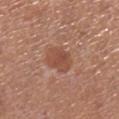* biopsy status · total-body-photography surveillance lesion; no biopsy
* illumination · white-light
* anatomic site · the left lower leg
* subject · female, approximately 65 years of age
* diameter · ~3 mm (longest diameter)
* image source · 15 mm crop, total-body photography